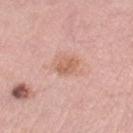<lesion>
  <biopsy_status>not biopsied; imaged during a skin examination</biopsy_status>
  <lighting>white-light</lighting>
  <site>left thigh</site>
  <lesion_size>
    <long_diameter_mm_approx>2.5</long_diameter_mm_approx>
  </lesion_size>
  <patient>
    <sex>female</sex>
    <age_approx>40</age_approx>
  </patient>
  <image>
    <source>total-body photography crop</source>
    <field_of_view_mm>15</field_of_view_mm>
  </image>
</lesion>